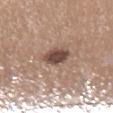Imaged during a routine full-body skin examination; the lesion was not biopsied and no histopathology is available.